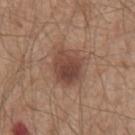Clinical impression:
The lesion was photographed on a routine skin check and not biopsied; there is no pathology result.
Acquisition and patient details:
The lesion is on the abdomen. A male patient about 65 years old. Cropped from a whole-body photographic skin survey; the tile spans about 15 mm. The lesion-visualizer software estimated a border-irregularity rating of about 2/10, a color-variation rating of about 5/10, and a peripheral color-asymmetry measure near 1.5.